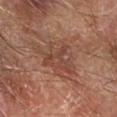The lesion is located on the arm. This is a cross-polarized tile. This image is a 15 mm lesion crop taken from a total-body photograph. About 5.5 mm across. The total-body-photography lesion software estimated a border-irregularity rating of about 8/10, a color-variation rating of about 4/10, and a peripheral color-asymmetry measure near 1.5. The analysis additionally found an automated nevus-likeness rating near 0 out of 100 and a detector confidence of about 70 out of 100 that the crop contains a lesion. A male subject, roughly 70 years of age.